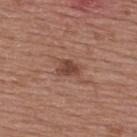biopsy status: no biopsy performed (imaged during a skin exam)
site: the upper back
size: about 2.5 mm
imaging modality: total-body-photography crop, ~15 mm field of view
patient: male, approximately 55 years of age
image-analysis metrics: an area of roughly 4.5 mm², an eccentricity of roughly 0.65, and a symmetry-axis asymmetry near 0.35; a lesion color around L≈44 a*≈21 b*≈26 in CIELAB and a lesion-to-skin contrast of about 8 (normalized; higher = more distinct)